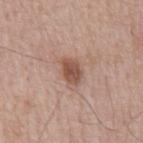  image:
    source: total-body photography crop
    field_of_view_mm: 15
  lesion_size:
    long_diameter_mm_approx: 3.0
  site: chest
  patient:
    sex: male
    age_approx: 65
  lighting: white-light